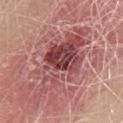* workup — total-body-photography surveillance lesion; no biopsy
* automated lesion analysis — an outline eccentricity of about 0.9 (0 = round, 1 = elongated) and two-axis asymmetry of about 0.35; an average lesion color of about L≈48 a*≈29 b*≈22 (CIELAB) and a lesion–skin lightness drop of about 13
* illumination — white-light
* patient — male, in their mid- to late 60s
* lesion size — about 11.5 mm
* anatomic site — the chest
* image — ~15 mm tile from a whole-body skin photo